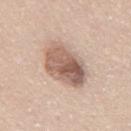<case>
  <biopsy_status>not biopsied; imaged during a skin examination</biopsy_status>
  <patient>
    <sex>male</sex>
    <age_approx>45</age_approx>
  </patient>
  <lighting>white-light</lighting>
  <image>
    <source>total-body photography crop</source>
    <field_of_view_mm>15</field_of_view_mm>
  </image>
  <lesion_size>
    <long_diameter_mm_approx>6.0</long_diameter_mm_approx>
  </lesion_size>
  <site>mid back</site>
</case>